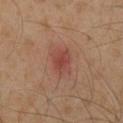Clinical impression:
The lesion was photographed on a routine skin check and not biopsied; there is no pathology result.
Acquisition and patient details:
The lesion is on the chest. The lesion's longest dimension is about 3 mm. A 15 mm close-up tile from a total-body photography series done for melanoma screening. Imaged with cross-polarized lighting. The patient is a male roughly 60 years of age.